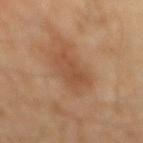The lesion was tiled from a total-body skin photograph and was not biopsied.
Automated tile analysis of the lesion measured an average lesion color of about L≈48 a*≈21 b*≈33 (CIELAB) and a lesion-to-skin contrast of about 6 (normalized; higher = more distinct). The software also gave a border-irregularity rating of about 3.5/10, internal color variation of about 2.5 on a 0–10 scale, and radial color variation of about 1.
A close-up tile cropped from a whole-body skin photograph, about 15 mm across.
The subject is a male aged 53–57.
From the back.
Measured at roughly 4.5 mm in maximum diameter.